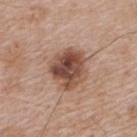Q: Was a biopsy performed?
A: imaged on a skin check; not biopsied
Q: What are the patient's age and sex?
A: male, aged 63–67
Q: What is the imaging modality?
A: ~15 mm tile from a whole-body skin photo
Q: What did automated image analysis measure?
A: a lesion area of about 15 mm² and two-axis asymmetry of about 0.25; a mean CIELAB color near L≈48 a*≈21 b*≈27, a lesion–skin lightness drop of about 15, and a normalized lesion–skin contrast near 10.5; a color-variation rating of about 7.5/10 and radial color variation of about 2.5
Q: Where on the body is the lesion?
A: the upper back